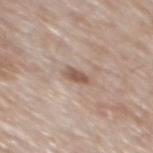The lesion was tiled from a total-body skin photograph and was not biopsied.
Located on the mid back.
Cropped from a total-body skin-imaging series; the visible field is about 15 mm.
A male subject, aged approximately 65.